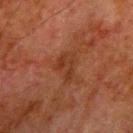biopsy status — no biopsy performed (imaged during a skin exam)
image source — 15 mm crop, total-body photography
TBP lesion metrics — an average lesion color of about L≈28 a*≈20 b*≈27 (CIELAB), roughly 5 lightness units darker than nearby skin, and a normalized lesion–skin contrast near 5.5; a classifier nevus-likeness of about 0/100 and a detector confidence of about 100 out of 100 that the crop contains a lesion
patient — male, aged 78–82
body site — the arm